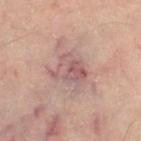Captured during whole-body skin photography for melanoma surveillance; the lesion was not biopsied. Automated image analysis of the tile measured a border-irregularity index near 3.5/10, a color-variation rating of about 6/10, and radial color variation of about 2. It also reported an automated nevus-likeness rating near 0 out of 100 and lesion-presence confidence of about 95/100. A female patient aged around 45. Approximately 4 mm at its widest. Cropped from a total-body skin-imaging series; the visible field is about 15 mm. From the left thigh. This is a cross-polarized tile.